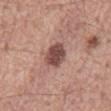Case summary:
– biopsy status: imaged on a skin check; not biopsied
– site: the back
– illumination: white-light illumination
– imaging modality: total-body-photography crop, ~15 mm field of view
– subject: male, aged approximately 55
– diameter: about 4 mm